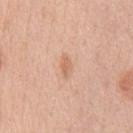<tbp_lesion>
<biopsy_status>not biopsied; imaged during a skin examination</biopsy_status>
<site>chest</site>
<image>
  <source>total-body photography crop</source>
  <field_of_view_mm>15</field_of_view_mm>
</image>
<patient>
  <sex>male</sex>
  <age_approx>50</age_approx>
</patient>
<lighting>white-light</lighting>
<lesion_size>
  <long_diameter_mm_approx>2.5</long_diameter_mm_approx>
</lesion_size>
</tbp_lesion>